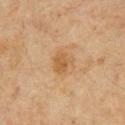Findings:
– acquisition — ~15 mm tile from a whole-body skin photo
– diameter — about 2.5 mm
– subject — male, approximately 65 years of age
– body site — the chest